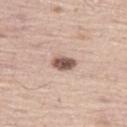Impression: No biopsy was performed on this lesion — it was imaged during a full skin examination and was not determined to be concerning. Clinical summary: The tile uses white-light illumination. From the left thigh. A region of skin cropped from a whole-body photographic capture, roughly 15 mm wide. The lesion's longest dimension is about 3 mm. The patient is a male approximately 65 years of age. Automated image analysis of the tile measured a mean CIELAB color near L≈54 a*≈18 b*≈24 and a lesion–skin lightness drop of about 18. The software also gave a border-irregularity rating of about 2/10 and a peripheral color-asymmetry measure near 1.5. And it measured an automated nevus-likeness rating near 100 out of 100 and a lesion-detection confidence of about 100/100.